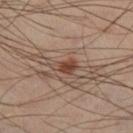The lesion was tiled from a total-body skin photograph and was not biopsied. The tile uses cross-polarized illumination. The patient is a male about 40 years old. About 3 mm across. This image is a 15 mm lesion crop taken from a total-body photograph. Automated tile analysis of the lesion measured a lesion area of about 4.5 mm². The analysis additionally found about 10 CIELAB-L* units darker than the surrounding skin and a normalized lesion–skin contrast near 8.5. The software also gave a classifier nevus-likeness of about 90/100 and a detector confidence of about 100 out of 100 that the crop contains a lesion. Located on the leg.